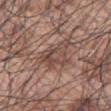Assessment:
This lesion was catalogued during total-body skin photography and was not selected for biopsy.
Acquisition and patient details:
This is a white-light tile. A close-up tile cropped from a whole-body skin photograph, about 15 mm across. Located on the chest. The patient is a male about 70 years old.